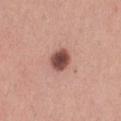Q: Was this lesion biopsied?
A: catalogued during a skin exam; not biopsied
Q: Where on the body is the lesion?
A: the leg
Q: What kind of image is this?
A: ~15 mm tile from a whole-body skin photo
Q: How large is the lesion?
A: about 3 mm
Q: What lighting was used for the tile?
A: white-light
Q: Patient demographics?
A: female, aged around 30
Q: Automated lesion metrics?
A: border irregularity of about 1 on a 0–10 scale, internal color variation of about 3.5 on a 0–10 scale, and a peripheral color-asymmetry measure near 0.5